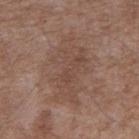Impression: Recorded during total-body skin imaging; not selected for excision or biopsy. Acquisition and patient details: The patient is a male about 50 years old. A 15 mm close-up tile from a total-body photography series done for melanoma screening. The tile uses white-light illumination. On the right upper arm. Longest diameter approximately 8 mm.